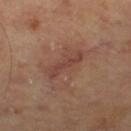<case>
<image>
  <source>total-body photography crop</source>
  <field_of_view_mm>15</field_of_view_mm>
</image>
<site>right leg</site>
<patient>
  <sex>female</sex>
  <age_approx>60</age_approx>
</patient>
</case>